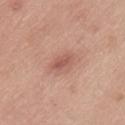Captured during whole-body skin photography for melanoma surveillance; the lesion was not biopsied.
The lesion is on the back.
A male subject, in their mid- to late 50s.
The tile uses white-light illumination.
Cropped from a total-body skin-imaging series; the visible field is about 15 mm.
Automated image analysis of the tile measured a lesion–skin lightness drop of about 9 and a normalized border contrast of about 6. The analysis additionally found a border-irregularity index near 2.5/10, internal color variation of about 2 on a 0–10 scale, and a peripheral color-asymmetry measure near 0.5.
The lesion's longest dimension is about 3 mm.